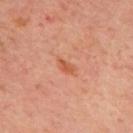Image and clinical context: Automated tile analysis of the lesion measured a border-irregularity index near 2.5/10 and a peripheral color-asymmetry measure near 0.5. It also reported an automated nevus-likeness rating near 75 out of 100. Cropped from a total-body skin-imaging series; the visible field is about 15 mm. The patient is a male aged approximately 65. Imaged with cross-polarized lighting. From the mid back. The recorded lesion diameter is about 2 mm.